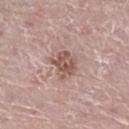Q: Where on the body is the lesion?
A: the right leg
Q: Who is the patient?
A: male, roughly 70 years of age
Q: Automated lesion metrics?
A: an area of roughly 7.5 mm², an eccentricity of roughly 0.45, and a symmetry-axis asymmetry near 0.25
Q: How large is the lesion?
A: ≈3 mm
Q: How was the tile lit?
A: white-light illumination
Q: What is the imaging modality?
A: total-body-photography crop, ~15 mm field of view